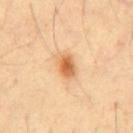biopsy_status: not biopsied; imaged during a skin examination
lesion_size:
  long_diameter_mm_approx: 3.0
lighting: cross-polarized
site: front of the torso
patient:
  sex: male
  age_approx: 60
image:
  source: total-body photography crop
  field_of_view_mm: 15